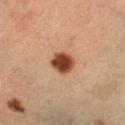{
  "biopsy_status": "not biopsied; imaged during a skin examination",
  "patient": {
    "sex": "female",
    "age_approx": 55
  },
  "site": "left lower leg",
  "image": {
    "source": "total-body photography crop",
    "field_of_view_mm": 15
  },
  "automated_metrics": {
    "area_mm2_approx": 6.5,
    "eccentricity": 0.3,
    "shape_asymmetry": 0.15,
    "border_irregularity_0_10": 1.0,
    "color_variation_0_10": 3.5,
    "peripheral_color_asymmetry": 1.0
  }
}